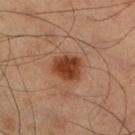Notes:
– follow-up: catalogued during a skin exam; not biopsied